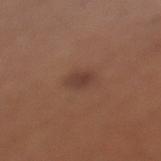Q: Was this lesion biopsied?
A: imaged on a skin check; not biopsied
Q: Who is the patient?
A: female, about 55 years old
Q: What is the anatomic site?
A: the left lower leg
Q: How was this image acquired?
A: total-body-photography crop, ~15 mm field of view
Q: Lesion size?
A: about 3 mm
Q: Illumination type?
A: white-light